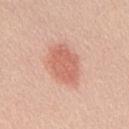Captured during whole-body skin photography for melanoma surveillance; the lesion was not biopsied. The tile uses white-light illumination. A 15 mm close-up tile from a total-body photography series done for melanoma screening. From the back. About 5 mm across. A male subject aged approximately 40. An algorithmic analysis of the crop reported a lesion–skin lightness drop of about 10 and a normalized lesion–skin contrast near 6.5. The analysis additionally found a nevus-likeness score of about 100/100.